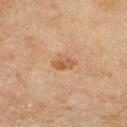{"biopsy_status": "not biopsied; imaged during a skin examination", "lesion_size": {"long_diameter_mm_approx": 2.5}, "patient": {"sex": "female", "age_approx": 60}, "automated_metrics": {"cielab_L": 52, "cielab_a": 20, "cielab_b": 35, "vs_skin_darker_L": 9.0, "vs_skin_contrast_norm": 7.0, "nevus_likeness_0_100": 35, "lesion_detection_confidence_0_100": 100}, "lighting": "cross-polarized", "image": {"source": "total-body photography crop", "field_of_view_mm": 15}, "site": "right thigh"}